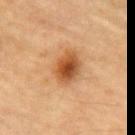<lesion>
<biopsy_status>not biopsied; imaged during a skin examination</biopsy_status>
<lesion_size>
  <long_diameter_mm_approx>4.0</long_diameter_mm_approx>
</lesion_size>
<image>
  <source>total-body photography crop</source>
  <field_of_view_mm>15</field_of_view_mm>
</image>
<patient>
  <sex>male</sex>
  <age_approx>85+</age_approx>
</patient>
<site>chest</site>
<automated_metrics>
  <area_mm2_approx>10.0</area_mm2_approx>
  <eccentricity>0.6</eccentricity>
  <shape_asymmetry>0.1</shape_asymmetry>
  <border_irregularity_0_10>1.5</border_irregularity_0_10>
  <color_variation_0_10>6.5</color_variation_0_10>
  <peripheral_color_asymmetry>2.0</peripheral_color_asymmetry>
  <lesion_detection_confidence_0_100>100</lesion_detection_confidence_0_100>
</automated_metrics>
</lesion>